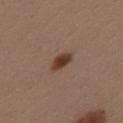workup = no biopsy performed (imaged during a skin exam) | automated metrics = an eccentricity of roughly 0.85 | anatomic site = the mid back | diameter = ≈3 mm | image source = ~15 mm tile from a whole-body skin photo | subject = male, aged 38–42 | tile lighting = white-light illumination.